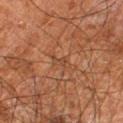Clinical impression:
No biopsy was performed on this lesion — it was imaged during a full skin examination and was not determined to be concerning.
Context:
Imaged with cross-polarized lighting. Longest diameter approximately 2.5 mm. On the right leg. This image is a 15 mm lesion crop taken from a total-body photograph. An algorithmic analysis of the crop reported a border-irregularity index near 4.5/10, a within-lesion color-variation index near 0/10, and radial color variation of about 0. It also reported a nevus-likeness score of about 0/100 and a detector confidence of about 55 out of 100 that the crop contains a lesion. The patient is a male aged approximately 60.